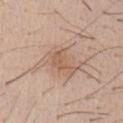Imaged during a routine full-body skin examination; the lesion was not biopsied and no histopathology is available.
A female patient aged 38–42.
Longest diameter approximately 3.5 mm.
The tile uses white-light illumination.
A lesion tile, about 15 mm wide, cut from a 3D total-body photograph.
The lesion-visualizer software estimated an average lesion color of about L≈58 a*≈18 b*≈30 (CIELAB) and about 7 CIELAB-L* units darker than the surrounding skin. And it measured a classifier nevus-likeness of about 5/100.
Located on the abdomen.